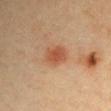biopsy status: no biopsy performed (imaged during a skin exam) | size: about 3 mm | subject: male, aged 38–42 | illumination: cross-polarized illumination | anatomic site: the left upper arm | TBP lesion metrics: a lesion color around L≈47 a*≈22 b*≈31 in CIELAB, a lesion–skin lightness drop of about 9, and a normalized border contrast of about 7 | image source: total-body-photography crop, ~15 mm field of view.